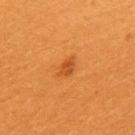Impression: The lesion was tiled from a total-body skin photograph and was not biopsied. Image and clinical context: Cropped from a total-body skin-imaging series; the visible field is about 15 mm. Located on the back. A female patient aged around 30. Automated tile analysis of the lesion measured roughly 9 lightness units darker than nearby skin and a lesion-to-skin contrast of about 6.5 (normalized; higher = more distinct). It also reported a within-lesion color-variation index near 2/10 and a peripheral color-asymmetry measure near 1. And it measured a classifier nevus-likeness of about 95/100. The recorded lesion diameter is about 2.5 mm. Imaged with cross-polarized lighting.